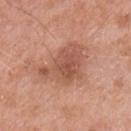This lesion was catalogued during total-body skin photography and was not selected for biopsy. The lesion is on the arm. The patient is a male about 55 years old. A lesion tile, about 15 mm wide, cut from a 3D total-body photograph. Measured at roughly 5.5 mm in maximum diameter.